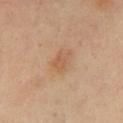biopsy status: catalogued during a skin exam; not biopsied
acquisition: ~15 mm tile from a whole-body skin photo
location: the front of the torso
subject: female, roughly 40 years of age